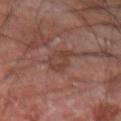The lesion was photographed on a routine skin check and not biopsied; there is no pathology result.
The lesion is on the right forearm.
Captured under cross-polarized illumination.
The lesion-visualizer software estimated a footprint of about 6 mm², an outline eccentricity of about 0.85 (0 = round, 1 = elongated), and a shape-asymmetry score of about 0.25 (0 = symmetric). It also reported a mean CIELAB color near L≈36 a*≈18 b*≈21 and roughly 6 lightness units darker than nearby skin. The software also gave a border-irregularity index near 3/10 and a color-variation rating of about 3/10. The software also gave an automated nevus-likeness rating near 0 out of 100 and a lesion-detection confidence of about 80/100.
The recorded lesion diameter is about 3.5 mm.
A 15 mm crop from a total-body photograph taken for skin-cancer surveillance.
The subject is a male roughly 60 years of age.